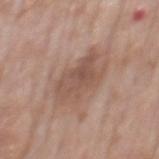Clinical summary:
A roughly 15 mm field-of-view crop from a total-body skin photograph. Approximately 5.5 mm at its widest. Located on the mid back. A male subject, roughly 75 years of age. Automated image analysis of the tile measured a mean CIELAB color near L≈52 a*≈18 b*≈26 and a lesion–skin lightness drop of about 9. The analysis additionally found a border-irregularity rating of about 4/10. It also reported a nevus-likeness score of about 0/100 and lesion-presence confidence of about 100/100. Captured under white-light illumination.